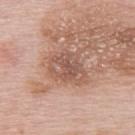Imaged during a routine full-body skin examination; the lesion was not biopsied and no histopathology is available.
The subject is a female aged 63 to 67.
The lesion is located on the upper back.
A close-up tile cropped from a whole-body skin photograph, about 15 mm across.